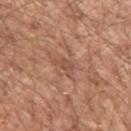Assessment: No biopsy was performed on this lesion — it was imaged during a full skin examination and was not determined to be concerning. Acquisition and patient details: The subject is a male in their mid- to late 60s. The lesion is located on the arm. Longest diameter approximately 3 mm. A region of skin cropped from a whole-body photographic capture, roughly 15 mm wide.